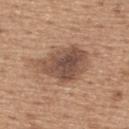Recorded during total-body skin imaging; not selected for excision or biopsy.
Imaged with white-light lighting.
Located on the upper back.
Cropped from a total-body skin-imaging series; the visible field is about 15 mm.
A male patient, roughly 65 years of age.
Automated image analysis of the tile measured an area of roughly 20 mm² and two-axis asymmetry of about 0.3. It also reported a border-irregularity index near 3.5/10.
About 7 mm across.